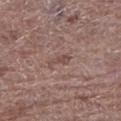Impression: Recorded during total-body skin imaging; not selected for excision or biopsy. Image and clinical context: A 15 mm close-up tile from a total-body photography series done for melanoma screening. A male subject, roughly 70 years of age. From the left lower leg. Approximately 3 mm at its widest.